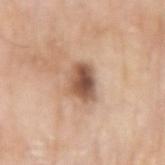Assessment: The lesion was tiled from a total-body skin photograph and was not biopsied. Acquisition and patient details: Automated image analysis of the tile measured a border-irregularity index near 2/10, a color-variation rating of about 6.5/10, and a peripheral color-asymmetry measure near 2. Cropped from a total-body skin-imaging series; the visible field is about 15 mm. The lesion is located on the right upper arm. The subject is a male aged approximately 80.